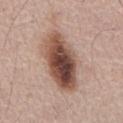{"biopsy_status": "not biopsied; imaged during a skin examination", "lighting": "white-light", "automated_metrics": {"cielab_L": 49, "cielab_a": 20, "cielab_b": 26, "border_irregularity_0_10": 2.0, "color_variation_0_10": 10.0, "peripheral_color_asymmetry": 3.5}, "site": "abdomen", "image": {"source": "total-body photography crop", "field_of_view_mm": 15}, "patient": {"sex": "male", "age_approx": 65}, "lesion_size": {"long_diameter_mm_approx": 8.0}}